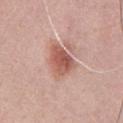Part of a total-body skin-imaging series; this lesion was reviewed on a skin check and was not flagged for biopsy. A region of skin cropped from a whole-body photographic capture, roughly 15 mm wide. On the front of the torso. The patient is a male about 60 years old.